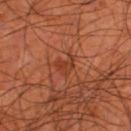Impression: No biopsy was performed on this lesion — it was imaged during a full skin examination and was not determined to be concerning. Acquisition and patient details: Approximately 2.5 mm at its widest. A region of skin cropped from a whole-body photographic capture, roughly 15 mm wide. A male subject, about 70 years old. On the leg. Captured under cross-polarized illumination.